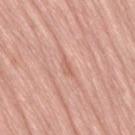The lesion was photographed on a routine skin check and not biopsied; there is no pathology result.
A female subject, aged 68–72.
This is a white-light tile.
Approximately 3 mm at its widest.
The lesion is located on the lower back.
Cropped from a total-body skin-imaging series; the visible field is about 15 mm.